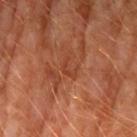Q: Was this lesion biopsied?
A: catalogued during a skin exam; not biopsied
Q: What are the patient's age and sex?
A: male, aged approximately 60
Q: What is the lesion's diameter?
A: ~2.5 mm (longest diameter)
Q: What is the imaging modality?
A: ~15 mm tile from a whole-body skin photo
Q: What did automated image analysis measure?
A: a lesion color around L≈39 a*≈27 b*≈33 in CIELAB, a lesion–skin lightness drop of about 6, and a normalized border contrast of about 5; a lesion-detection confidence of about 90/100
Q: What is the anatomic site?
A: the arm